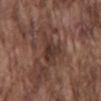Imaged during a routine full-body skin examination; the lesion was not biopsied and no histopathology is available.
Imaged with white-light lighting.
A lesion tile, about 15 mm wide, cut from a 3D total-body photograph.
From the mid back.
A male patient aged 73 to 77.
The lesion's longest dimension is about 4.5 mm.